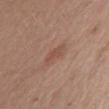{
  "biopsy_status": "not biopsied; imaged during a skin examination",
  "site": "left upper arm",
  "lighting": "white-light",
  "lesion_size": {
    "long_diameter_mm_approx": 2.5
  },
  "patient": {
    "sex": "female",
    "age_approx": 50
  },
  "image": {
    "source": "total-body photography crop",
    "field_of_view_mm": 15
  }
}